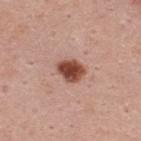follow-up: no biopsy performed (imaged during a skin exam); subject: male, aged 43–47; image source: 15 mm crop, total-body photography; size: ~3 mm (longest diameter); body site: the upper back; illumination: white-light illumination.